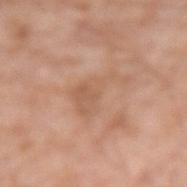{"biopsy_status": "not biopsied; imaged during a skin examination", "site": "right forearm", "image": {"source": "total-body photography crop", "field_of_view_mm": 15}, "patient": {"sex": "male", "age_approx": 80}, "automated_metrics": {"area_mm2_approx": 10.0, "eccentricity": 0.85, "shape_asymmetry": 0.65, "nevus_likeness_0_100": 0, "lesion_detection_confidence_0_100": 100}}